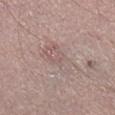Imaged with white-light lighting. Cropped from a whole-body photographic skin survey; the tile spans about 15 mm. The lesion's longest dimension is about 5.5 mm. Located on the leg. The subject is a male in their mid- to late 40s. Automated image analysis of the tile measured an area of roughly 13 mm² and an eccentricity of roughly 0.85. And it measured an average lesion color of about L≈58 a*≈16 b*≈19 (CIELAB), roughly 5 lightness units darker than nearby skin, and a normalized border contrast of about 3.5.